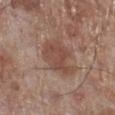Q: Was this lesion biopsied?
A: no biopsy performed (imaged during a skin exam)
Q: Who is the patient?
A: male, aged around 70
Q: How large is the lesion?
A: ≈4.5 mm
Q: What is the anatomic site?
A: the right lower leg
Q: What is the imaging modality?
A: ~15 mm tile from a whole-body skin photo
Q: What did automated image analysis measure?
A: an area of roughly 12 mm², a shape eccentricity near 0.6, and two-axis asymmetry of about 0.35; a border-irregularity index near 4/10 and a color-variation rating of about 2.5/10
Q: How was the tile lit?
A: white-light illumination